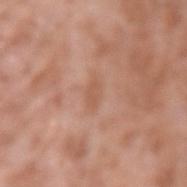No biopsy was performed on this lesion — it was imaged during a full skin examination and was not determined to be concerning. Measured at roughly 2.5 mm in maximum diameter. A region of skin cropped from a whole-body photographic capture, roughly 15 mm wide. Captured under white-light illumination. The subject is a male aged approximately 60. From the left upper arm. An algorithmic analysis of the crop reported border irregularity of about 2.5 on a 0–10 scale and internal color variation of about 0.5 on a 0–10 scale. It also reported a nevus-likeness score of about 0/100 and lesion-presence confidence of about 100/100.